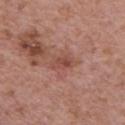Case summary:
* workup — catalogued during a skin exam; not biopsied
* subject — male, aged 68–72
* automated metrics — a lesion area of about 5.5 mm² and a symmetry-axis asymmetry near 0.25; a within-lesion color-variation index near 4/10 and a peripheral color-asymmetry measure near 1.5; a classifier nevus-likeness of about 0/100 and a detector confidence of about 100 out of 100 that the crop contains a lesion
* body site — the chest
* size — ~3.5 mm (longest diameter)
* image — ~15 mm crop, total-body skin-cancer survey